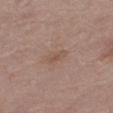The patient is a female aged around 55.
The tile uses white-light illumination.
From the right thigh.
A lesion tile, about 15 mm wide, cut from a 3D total-body photograph.
The recorded lesion diameter is about 3 mm.
Automated tile analysis of the lesion measured an average lesion color of about L≈52 a*≈17 b*≈26 (CIELAB), about 6 CIELAB-L* units darker than the surrounding skin, and a normalized lesion–skin contrast near 5. The software also gave a nevus-likeness score of about 0/100 and a lesion-detection confidence of about 100/100.